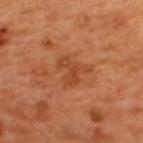Clinical impression:
No biopsy was performed on this lesion — it was imaged during a full skin examination and was not determined to be concerning.
Background:
Approximately 3.5 mm at its widest. A lesion tile, about 15 mm wide, cut from a 3D total-body photograph. A male subject in their 50s. On the upper back.